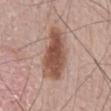Recorded during total-body skin imaging; not selected for excision or biopsy. This is a white-light tile. A region of skin cropped from a whole-body photographic capture, roughly 15 mm wide. The lesion's longest dimension is about 6.5 mm. The subject is a male aged 53 to 57.